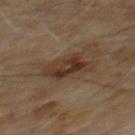follow-up: catalogued during a skin exam; not biopsied
image: 15 mm crop, total-body photography
patient: male, roughly 60 years of age
site: the chest
lesion diameter: ~5 mm (longest diameter)